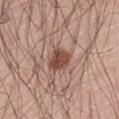* biopsy status: no biopsy performed (imaged during a skin exam)
* image: total-body-photography crop, ~15 mm field of view
* site: the abdomen
* lighting: white-light illumination
* automated metrics: a lesion area of about 7 mm²; a lesion color around L≈48 a*≈22 b*≈26 in CIELAB, roughly 13 lightness units darker than nearby skin, and a normalized border contrast of about 9
* subject: male, roughly 60 years of age
* size: ~3 mm (longest diameter)